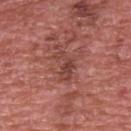Captured during whole-body skin photography for melanoma surveillance; the lesion was not biopsied.
From the upper back.
A close-up tile cropped from a whole-body skin photograph, about 15 mm across.
The patient is a male aged around 70.
Measured at roughly 3.5 mm in maximum diameter.